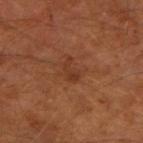Findings:
• notes — imaged on a skin check; not biopsied
• body site — the right upper arm
• image source — total-body-photography crop, ~15 mm field of view
• patient — male, approximately 60 years of age